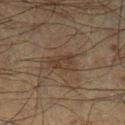Imaged during a routine full-body skin examination; the lesion was not biopsied and no histopathology is available.